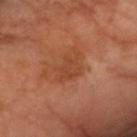Recorded during total-body skin imaging; not selected for excision or biopsy. Located on the right forearm. A lesion tile, about 15 mm wide, cut from a 3D total-body photograph. The lesion-visualizer software estimated an average lesion color of about L≈43 a*≈25 b*≈33 (CIELAB) and a normalized lesion–skin contrast near 5. The analysis additionally found border irregularity of about 4 on a 0–10 scale, a within-lesion color-variation index near 2/10, and a peripheral color-asymmetry measure near 0.5. The analysis additionally found an automated nevus-likeness rating near 0 out of 100 and a detector confidence of about 100 out of 100 that the crop contains a lesion. Imaged with cross-polarized lighting. Longest diameter approximately 4 mm. The subject is a female in their 70s.